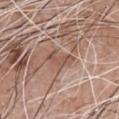Impression: This lesion was catalogued during total-body skin photography and was not selected for biopsy. Image and clinical context: On the chest. This image is a 15 mm lesion crop taken from a total-body photograph. Longest diameter approximately 3.5 mm. A male patient about 60 years old.